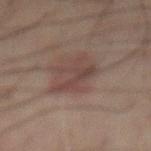The lesion was tiled from a total-body skin photograph and was not biopsied.
Imaged with cross-polarized lighting.
The lesion-visualizer software estimated an area of roughly 9 mm², an eccentricity of roughly 0.8, and a shape-asymmetry score of about 0.6 (0 = symmetric). It also reported a mean CIELAB color near L≈34 a*≈14 b*≈17, a lesion–skin lightness drop of about 6, and a normalized border contrast of about 6. The analysis additionally found a border-irregularity rating of about 8.5/10 and radial color variation of about 1. And it measured a detector confidence of about 100 out of 100 that the crop contains a lesion.
A male subject roughly 70 years of age.
The lesion's longest dimension is about 5 mm.
On the mid back.
A roughly 15 mm field-of-view crop from a total-body skin photograph.